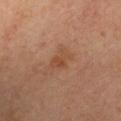Assessment:
Part of a total-body skin-imaging series; this lesion was reviewed on a skin check and was not flagged for biopsy.
Background:
Cropped from a whole-body photographic skin survey; the tile spans about 15 mm. This is a cross-polarized tile. An algorithmic analysis of the crop reported an area of roughly 5 mm², an outline eccentricity of about 0.8 (0 = round, 1 = elongated), and two-axis asymmetry of about 0.3. And it measured a lesion–skin lightness drop of about 6. It also reported border irregularity of about 4 on a 0–10 scale and internal color variation of about 2 on a 0–10 scale. And it measured a classifier nevus-likeness of about 0/100 and lesion-presence confidence of about 100/100. From the chest. A female subject, aged around 35. The lesion's longest dimension is about 3.5 mm.